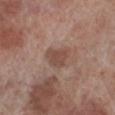Context:
Cropped from a whole-body photographic skin survey; the tile spans about 15 mm. The recorded lesion diameter is about 3.5 mm. From the left lower leg. The subject is a male aged 68–72.